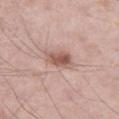Findings:
• workup — catalogued during a skin exam; not biopsied
• site — the right thigh
• lesion size — about 3 mm
• subject — male, aged 53–57
• image — ~15 mm tile from a whole-body skin photo